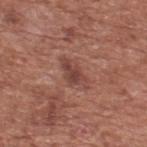Case summary:
– follow-up: imaged on a skin check; not biopsied
– TBP lesion metrics: a nevus-likeness score of about 0/100 and a detector confidence of about 100 out of 100 that the crop contains a lesion
– imaging modality: ~15 mm tile from a whole-body skin photo
– patient: male, in their mid-70s
– site: the back
– diameter: ≈4 mm
– illumination: white-light